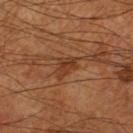- workup: no biopsy performed (imaged during a skin exam)
- imaging modality: total-body-photography crop, ~15 mm field of view
- subject: male, aged approximately 65
- location: the left leg